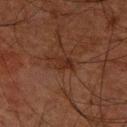Q: Was a biopsy performed?
A: imaged on a skin check; not biopsied
Q: What kind of image is this?
A: ~15 mm crop, total-body skin-cancer survey
Q: Where on the body is the lesion?
A: the left forearm
Q: Automated lesion metrics?
A: an area of roughly 4.5 mm² and a shape-asymmetry score of about 0.4 (0 = symmetric)
Q: Lesion size?
A: ≈3 mm
Q: Who is the patient?
A: male, aged around 80